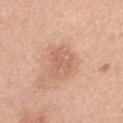<case>
  <biopsy_status>not biopsied; imaged during a skin examination</biopsy_status>
  <lesion_size>
    <long_diameter_mm_approx>3.5</long_diameter_mm_approx>
  </lesion_size>
  <image>
    <source>total-body photography crop</source>
    <field_of_view_mm>15</field_of_view_mm>
  </image>
  <patient>
    <sex>female</sex>
    <age_approx>45</age_approx>
  </patient>
  <site>right upper arm</site>
  <lighting>white-light</lighting>
</case>